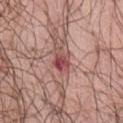The lesion was photographed on a routine skin check and not biopsied; there is no pathology result.
A lesion tile, about 15 mm wide, cut from a 3D total-body photograph.
The recorded lesion diameter is about 2.5 mm.
The lesion is on the abdomen.
Imaged with white-light lighting.
A female patient aged approximately 55.
The lesion-visualizer software estimated an area of roughly 4 mm², an eccentricity of roughly 0.65, and a symmetry-axis asymmetry near 0.35. And it measured an average lesion color of about L≈48 a*≈27 b*≈21 (CIELAB), a lesion–skin lightness drop of about 13, and a normalized border contrast of about 9. And it measured border irregularity of about 3 on a 0–10 scale and a within-lesion color-variation index near 6/10. And it measured an automated nevus-likeness rating near 0 out of 100 and lesion-presence confidence of about 100/100.